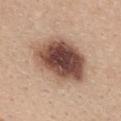Impression: No biopsy was performed on this lesion — it was imaged during a full skin examination and was not determined to be concerning. Image and clinical context: Located on the upper back. Imaged with white-light lighting. A 15 mm crop from a total-body photograph taken for skin-cancer surveillance. Approximately 8 mm at its widest. A female patient, in their mid- to late 20s.